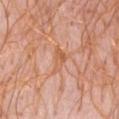Impression: Part of a total-body skin-imaging series; this lesion was reviewed on a skin check and was not flagged for biopsy. Background: A female patient about 45 years old. Cropped from a whole-body photographic skin survey; the tile spans about 15 mm. On the abdomen. The tile uses white-light illumination.